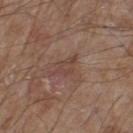Clinical summary: From the leg. The tile uses white-light illumination. A 15 mm close-up extracted from a 3D total-body photography capture. The patient is a male roughly 80 years of age. The lesion's longest dimension is about 3 mm. An algorithmic analysis of the crop reported an average lesion color of about L≈43 a*≈18 b*≈24 (CIELAB) and a normalized lesion–skin contrast near 5.5. The software also gave a border-irregularity rating of about 7.5/10 and radial color variation of about 0.